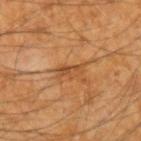Findings:
* notes · imaged on a skin check; not biopsied
* location · the left forearm
* image · ~15 mm crop, total-body skin-cancer survey
* diameter · about 3 mm
* subject · male, about 60 years old
* illumination · cross-polarized